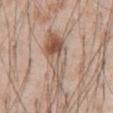{
  "site": "abdomen",
  "lesion_size": {
    "long_diameter_mm_approx": 8.0
  },
  "image": {
    "source": "total-body photography crop",
    "field_of_view_mm": 15
  },
  "lighting": "white-light",
  "patient": {
    "sex": "male",
    "age_approx": 55
  },
  "automated_metrics": {
    "border_irregularity_0_10": 5.5,
    "color_variation_0_10": 10.0,
    "nevus_likeness_0_100": 90
  }
}